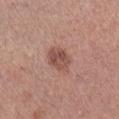workup: total-body-photography surveillance lesion; no biopsy
anatomic site: the right lower leg
image: 15 mm crop, total-body photography
subject: female, approximately 40 years of age